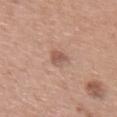Captured during whole-body skin photography for melanoma surveillance; the lesion was not biopsied.
A female subject approximately 60 years of age.
The lesion is on the upper back.
A 15 mm close-up extracted from a 3D total-body photography capture.